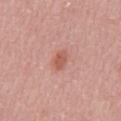biopsy status: total-body-photography surveillance lesion; no biopsy
size: about 2.5 mm
subject: male, aged 48–52
location: the abdomen
automated lesion analysis: an outline eccentricity of about 0.75 (0 = round, 1 = elongated) and two-axis asymmetry of about 0.2; an average lesion color of about L≈57 a*≈27 b*≈29 (CIELAB)
acquisition: 15 mm crop, total-body photography